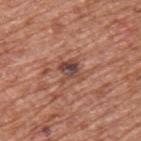biopsy_status: not biopsied; imaged during a skin examination
automated_metrics:
  vs_skin_darker_L: 11.0
  border_irregularity_0_10: 3.5
  color_variation_0_10: 7.5
  peripheral_color_asymmetry: 2.5
patient:
  sex: male
  age_approx: 65
site: upper back
lighting: white-light
image:
  source: total-body photography crop
  field_of_view_mm: 15
lesion_size:
  long_diameter_mm_approx: 2.5